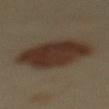notes — catalogued during a skin exam; not biopsied
site — the mid back
lesion size — ~7 mm (longest diameter)
automated lesion analysis — a footprint of about 31 mm², a shape eccentricity near 0.6, and two-axis asymmetry of about 0.15; a mean CIELAB color near L≈32 a*≈14 b*≈24, about 12 CIELAB-L* units darker than the surrounding skin, and a normalized lesion–skin contrast near 11
subject — female, in their 40s
imaging modality — ~15 mm tile from a whole-body skin photo